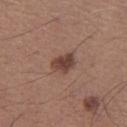{
  "biopsy_status": "not biopsied; imaged during a skin examination"
}